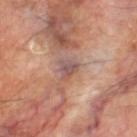Impression: Recorded during total-body skin imaging; not selected for excision or biopsy. Clinical summary: About 2.5 mm across. Captured under cross-polarized illumination. From the leg. The total-body-photography lesion software estimated a lesion color around L≈51 a*≈19 b*≈21 in CIELAB, about 9 CIELAB-L* units darker than the surrounding skin, and a normalized lesion–skin contrast near 7.5. It also reported a border-irregularity rating of about 3.5/10 and internal color variation of about 3 on a 0–10 scale. A close-up tile cropped from a whole-body skin photograph, about 15 mm across. A male subject, roughly 70 years of age.